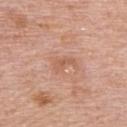biopsy_status: not biopsied; imaged during a skin examination
patient:
  sex: male
  age_approx: 75
image:
  source: total-body photography crop
  field_of_view_mm: 15
lighting: white-light
site: upper back